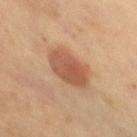Assessment: Part of a total-body skin-imaging series; this lesion was reviewed on a skin check and was not flagged for biopsy. Image and clinical context: A region of skin cropped from a whole-body photographic capture, roughly 15 mm wide. About 4.5 mm across. The lesion-visualizer software estimated internal color variation of about 3 on a 0–10 scale and peripheral color asymmetry of about 1. Captured under cross-polarized illumination. From the chest. The subject is a female aged 58–62.